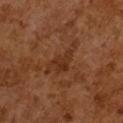Q: Was a biopsy performed?
A: total-body-photography surveillance lesion; no biopsy
Q: Lesion size?
A: ~5.5 mm (longest diameter)
Q: What is the imaging modality?
A: ~15 mm crop, total-body skin-cancer survey
Q: Illumination type?
A: cross-polarized illumination
Q: What are the patient's age and sex?
A: male, approximately 65 years of age